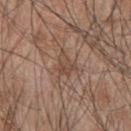biopsy status: no biopsy performed (imaged during a skin exam); image source: ~15 mm crop, total-body skin-cancer survey; anatomic site: the upper back; tile lighting: white-light illumination; size: ≈2.5 mm; subject: male, aged around 45.